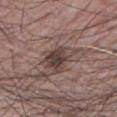Assessment:
Part of a total-body skin-imaging series; this lesion was reviewed on a skin check and was not flagged for biopsy.
Clinical summary:
A 15 mm crop from a total-body photograph taken for skin-cancer surveillance. Approximately 4.5 mm at its widest. A male subject in their 60s. Located on the left lower leg. This is a white-light tile.